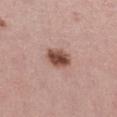A 15 mm crop from a total-body photograph taken for skin-cancer surveillance. The tile uses white-light illumination. The patient is a female roughly 45 years of age. The lesion is on the left thigh. The total-body-photography lesion software estimated a footprint of about 6.5 mm², an eccentricity of roughly 0.55, and a symmetry-axis asymmetry near 0.15. And it measured a lesion color around L≈49 a*≈22 b*≈26 in CIELAB and a lesion-to-skin contrast of about 11 (normalized; higher = more distinct). The analysis additionally found lesion-presence confidence of about 100/100. Longest diameter approximately 3 mm.